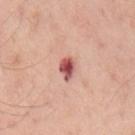| feature | finding |
|---|---|
| follow-up | no biopsy performed (imaged during a skin exam) |
| size | ≈2.5 mm |
| site | the mid back |
| subject | male, aged 53 to 57 |
| imaging modality | ~15 mm tile from a whole-body skin photo |
| TBP lesion metrics | an area of roughly 4 mm² and an outline eccentricity of about 0.7 (0 = round, 1 = elongated); a lesion color around L≈53 a*≈31 b*≈24 in CIELAB, a lesion–skin lightness drop of about 18, and a normalized lesion–skin contrast near 11.5; a classifier nevus-likeness of about 5/100 |